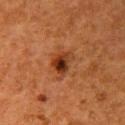workup: total-body-photography surveillance lesion; no biopsy
patient: female, aged approximately 50
acquisition: 15 mm crop, total-body photography
lesion diameter: ≈3 mm
anatomic site: the right upper arm
lighting: cross-polarized